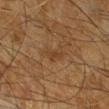<tbp_lesion>
<biopsy_status>not biopsied; imaged during a skin examination</biopsy_status>
<lesion_size>
  <long_diameter_mm_approx>2.5</long_diameter_mm_approx>
</lesion_size>
<image>
  <source>total-body photography crop</source>
  <field_of_view_mm>15</field_of_view_mm>
</image>
<site>leg</site>
<automated_metrics>
  <area_mm2_approx>2.5</area_mm2_approx>
  <eccentricity>0.9</eccentricity>
  <shape_asymmetry>0.35</shape_asymmetry>
  <cielab_L>31</cielab_L>
  <cielab_a>16</cielab_a>
  <cielab_b>28</cielab_b>
  <vs_skin_darker_L>5.0</vs_skin_darker_L>
  <vs_skin_contrast_norm>5.5</vs_skin_contrast_norm>
</automated_metrics>
<patient>
  <sex>male</sex>
  <age_approx>65</age_approx>
</patient>
<lighting>cross-polarized</lighting>
</tbp_lesion>